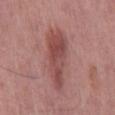follow-up = imaged on a skin check; not biopsied | size = about 7.5 mm | TBP lesion metrics = a lesion color around L≈48 a*≈25 b*≈22 in CIELAB, about 10 CIELAB-L* units darker than the surrounding skin, and a normalized border contrast of about 7.5; a border-irregularity rating of about 3.5/10, a color-variation rating of about 4/10, and peripheral color asymmetry of about 1.5; a nevus-likeness score of about 15/100 and a detector confidence of about 100 out of 100 that the crop contains a lesion | anatomic site = the mid back | image = total-body-photography crop, ~15 mm field of view | tile lighting = white-light | subject = male, aged 58–62.